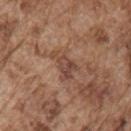{"biopsy_status": "not biopsied; imaged during a skin examination", "lighting": "white-light", "patient": {"sex": "male", "age_approx": 75}, "site": "right upper arm", "lesion_size": {"long_diameter_mm_approx": 4.0}, "image": {"source": "total-body photography crop", "field_of_view_mm": 15}}